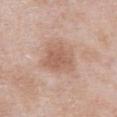Part of a total-body skin-imaging series; this lesion was reviewed on a skin check and was not flagged for biopsy.
The patient is a male aged approximately 55.
The total-body-photography lesion software estimated a lesion area of about 14 mm² and an outline eccentricity of about 0.5 (0 = round, 1 = elongated). It also reported a border-irregularity index near 2.5/10, a within-lesion color-variation index near 3/10, and a peripheral color-asymmetry measure near 1.
Captured under white-light illumination.
A roughly 15 mm field-of-view crop from a total-body skin photograph.
From the abdomen.